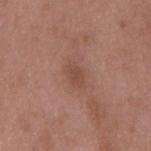{"biopsy_status": "not biopsied; imaged during a skin examination", "site": "mid back", "lighting": "white-light", "automated_metrics": {"eccentricity": 0.8, "shape_asymmetry": 0.2, "cielab_L": 48, "cielab_a": 22, "cielab_b": 27, "vs_skin_contrast_norm": 5.5}, "image": {"source": "total-body photography crop", "field_of_view_mm": 15}, "patient": {"sex": "male", "age_approx": 50}}